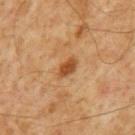Findings:
- notes — total-body-photography surveillance lesion; no biopsy
- image-analysis metrics — an average lesion color of about L≈46 a*≈22 b*≈38 (CIELAB), roughly 11 lightness units darker than nearby skin, and a lesion-to-skin contrast of about 9 (normalized; higher = more distinct); border irregularity of about 2.5 on a 0–10 scale and a peripheral color-asymmetry measure near 0.5
- image source — ~15 mm tile from a whole-body skin photo
- lighting — cross-polarized
- location — the mid back
- subject — male, roughly 60 years of age